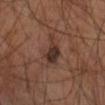The patient is aged approximately 55.
This image is a 15 mm lesion crop taken from a total-body photograph.
Located on the right forearm.
On excision, pathology confirmed an atypical melanocytic neoplasm, classified as a borderline lesion of uncertain malignant potential.